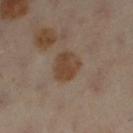Background: Captured under cross-polarized illumination. Longest diameter approximately 3.5 mm. A region of skin cropped from a whole-body photographic capture, roughly 15 mm wide. On the right leg. A female patient, about 60 years old. An algorithmic analysis of the crop reported a lesion area of about 9 mm², a shape eccentricity near 0.45, and a symmetry-axis asymmetry near 0.2. And it measured a border-irregularity index near 2/10 and internal color variation of about 2.5 on a 0–10 scale. The software also gave a classifier nevus-likeness of about 35/100 and lesion-presence confidence of about 100/100.